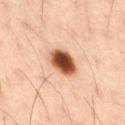Context:
The lesion is located on the right thigh. A male subject, aged 33–37. The tile uses cross-polarized illumination. A lesion tile, about 15 mm wide, cut from a 3D total-body photograph. Approximately 4 mm at its widest.